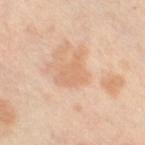Impression:
Captured during whole-body skin photography for melanoma surveillance; the lesion was not biopsied.
Context:
A 15 mm close-up extracted from a 3D total-body photography capture. Captured under cross-polarized illumination. The subject is a female aged around 50. Measured at roughly 4 mm in maximum diameter. The total-body-photography lesion software estimated a lesion area of about 7 mm² and a symmetry-axis asymmetry near 0.45. The analysis additionally found a border-irregularity index near 5/10, a color-variation rating of about 1.5/10, and a peripheral color-asymmetry measure near 0.5. The analysis additionally found an automated nevus-likeness rating near 0 out of 100. Located on the right thigh.